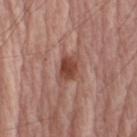Captured during whole-body skin photography for melanoma surveillance; the lesion was not biopsied.
The lesion is located on the right upper arm.
A roughly 15 mm field-of-view crop from a total-body skin photograph.
A male patient, aged 63–67.